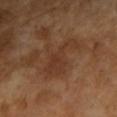The lesion was photographed on a routine skin check and not biopsied; there is no pathology result. A female patient, aged 68–72. Located on the left forearm. A 15 mm close-up extracted from a 3D total-body photography capture. The tile uses cross-polarized illumination. The lesion-visualizer software estimated border irregularity of about 9 on a 0–10 scale, a within-lesion color-variation index near 3/10, and peripheral color asymmetry of about 1.